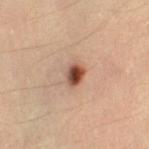{
  "biopsy_status": "not biopsied; imaged during a skin examination",
  "site": "left thigh",
  "image": {
    "source": "total-body photography crop",
    "field_of_view_mm": 15
  },
  "automated_metrics": {
    "cielab_L": 49,
    "cielab_a": 23,
    "cielab_b": 30,
    "vs_skin_darker_L": 17.0,
    "vs_skin_contrast_norm": 12.0,
    "border_irregularity_0_10": 1.5,
    "color_variation_0_10": 9.5,
    "peripheral_color_asymmetry": 3.0,
    "lesion_detection_confidence_0_100": 100
  },
  "lighting": "cross-polarized",
  "patient": {
    "sex": "female",
    "age_approx": 35
  }
}